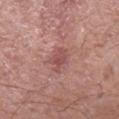Part of a total-body skin-imaging series; this lesion was reviewed on a skin check and was not flagged for biopsy. From the left forearm. Cropped from a whole-body photographic skin survey; the tile spans about 15 mm. The subject is a male aged 58 to 62. About 3 mm across.